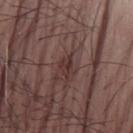{
  "biopsy_status": "not biopsied; imaged during a skin examination",
  "lesion_size": {
    "long_diameter_mm_approx": 2.5
  },
  "patient": {
    "sex": "male",
    "age_approx": 70
  },
  "site": "right forearm",
  "lighting": "white-light",
  "image": {
    "source": "total-body photography crop",
    "field_of_view_mm": 15
  }
}